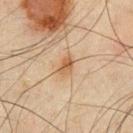Notes:
– workup: no biopsy performed (imaged during a skin exam)
– diameter: about 3 mm
– anatomic site: the chest
– patient: male, aged 43 to 47
– illumination: cross-polarized illumination
– image: ~15 mm tile from a whole-body skin photo